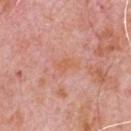Imaged during a routine full-body skin examination; the lesion was not biopsied and no histopathology is available. The tile uses white-light illumination. A lesion tile, about 15 mm wide, cut from a 3D total-body photograph. The recorded lesion diameter is about 3 mm. Located on the front of the torso. A male patient, roughly 80 years of age.